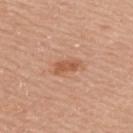Findings:
- workup — no biopsy performed (imaged during a skin exam)
- lesion diameter — ≈3 mm
- automated metrics — a footprint of about 3.5 mm², an eccentricity of roughly 0.9, and two-axis asymmetry of about 0.25; a lesion color around L≈56 a*≈25 b*≈35 in CIELAB, roughly 10 lightness units darker than nearby skin, and a normalized border contrast of about 7; a border-irregularity index near 2.5/10, a within-lesion color-variation index near 2.5/10, and radial color variation of about 1
- lighting — white-light illumination
- subject — female, about 65 years old
- body site — the upper back
- imaging modality — 15 mm crop, total-body photography